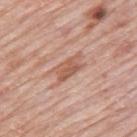Assessment: The lesion was tiled from a total-body skin photograph and was not biopsied. Context: A lesion tile, about 15 mm wide, cut from a 3D total-body photograph. From the mid back. About 4 mm across. A male subject in their 80s. Imaged with white-light lighting.